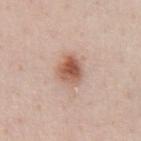Impression: Recorded during total-body skin imaging; not selected for excision or biopsy. Background: The subject is a male about 55 years old. Captured under white-light illumination. Measured at roughly 3.5 mm in maximum diameter. From the front of the torso. A 15 mm close-up extracted from a 3D total-body photography capture.